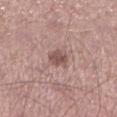| feature | finding |
|---|---|
| biopsy status | catalogued during a skin exam; not biopsied |
| automated metrics | an automated nevus-likeness rating near 45 out of 100 and a lesion-detection confidence of about 100/100 |
| illumination | white-light |
| lesion size | about 2.5 mm |
| acquisition | ~15 mm tile from a whole-body skin photo |
| site | the right lower leg |
| patient | male, roughly 40 years of age |